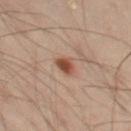No biopsy was performed on this lesion — it was imaged during a full skin examination and was not determined to be concerning.
The lesion's longest dimension is about 2.5 mm.
A male patient, approximately 50 years of age.
On the left thigh.
A 15 mm close-up tile from a total-body photography series done for melanoma screening.
The tile uses cross-polarized illumination.